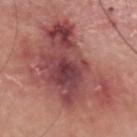Notes:
* biopsy status — total-body-photography surveillance lesion; no biopsy
* tile lighting — white-light
* subject — male, in their mid-60s
* body site — the head or neck
* diameter — ≈10 mm
* imaging modality — ~15 mm tile from a whole-body skin photo